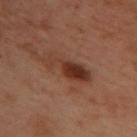Part of a total-body skin-imaging series; this lesion was reviewed on a skin check and was not flagged for biopsy. The tile uses cross-polarized illumination. The recorded lesion diameter is about 6.5 mm. Automated image analysis of the tile measured roughly 8 lightness units darker than nearby skin. It also reported border irregularity of about 4 on a 0–10 scale, internal color variation of about 5 on a 0–10 scale, and peripheral color asymmetry of about 1.5. The lesion is on the upper back. Cropped from a whole-body photographic skin survey; the tile spans about 15 mm. A female patient, aged 53 to 57.